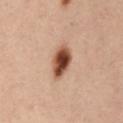Clinical impression: Part of a total-body skin-imaging series; this lesion was reviewed on a skin check and was not flagged for biopsy. Image and clinical context: The recorded lesion diameter is about 4 mm. A roughly 15 mm field-of-view crop from a total-body skin photograph. The subject is a female aged approximately 55. Located on the front of the torso. Imaged with cross-polarized lighting.